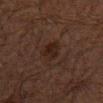Part of a total-body skin-imaging series; this lesion was reviewed on a skin check and was not flagged for biopsy. A 15 mm close-up extracted from a 3D total-body photography capture. A male subject, aged 58 to 62. From the mid back. This is a cross-polarized tile. Measured at roughly 3.5 mm in maximum diameter.